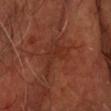Q: Was this lesion biopsied?
A: catalogued during a skin exam; not biopsied
Q: Automated lesion metrics?
A: a color-variation rating of about 4/10; an automated nevus-likeness rating near 0 out of 100 and a lesion-detection confidence of about 90/100
Q: How large is the lesion?
A: ~4.5 mm (longest diameter)
Q: Lesion location?
A: the head or neck
Q: How was this image acquired?
A: total-body-photography crop, ~15 mm field of view
Q: Who is the patient?
A: male, aged 63–67